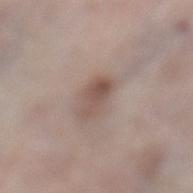Context: A lesion tile, about 15 mm wide, cut from a 3D total-body photograph. The subject is a female in their mid- to late 60s. An algorithmic analysis of the crop reported an area of roughly 6 mm² and two-axis asymmetry of about 0.25. It also reported about 10 CIELAB-L* units darker than the surrounding skin and a lesion-to-skin contrast of about 7 (normalized; higher = more distinct). Located on the left lower leg. Captured under white-light illumination.